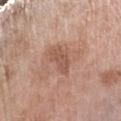{
  "biopsy_status": "not biopsied; imaged during a skin examination",
  "automated_metrics": {
    "border_irregularity_0_10": 4.0,
    "color_variation_0_10": 1.0,
    "peripheral_color_asymmetry": 0.5,
    "lesion_detection_confidence_0_100": 100
  },
  "lesion_size": {
    "long_diameter_mm_approx": 4.0
  },
  "site": "arm",
  "image": {
    "source": "total-body photography crop",
    "field_of_view_mm": 15
  },
  "lighting": "white-light",
  "patient": {
    "sex": "female",
    "age_approx": 75
  }
}